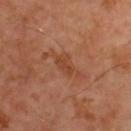<record>
  <biopsy_status>not biopsied; imaged during a skin examination</biopsy_status>
  <site>upper back</site>
  <automated_metrics>
    <area_mm2_approx>3.0</area_mm2_approx>
    <eccentricity>0.8</eccentricity>
    <shape_asymmetry>0.35</shape_asymmetry>
    <cielab_L>43</cielab_L>
    <cielab_a>25</cielab_a>
    <cielab_b>34</cielab_b>
    <vs_skin_darker_L>7.0</vs_skin_darker_L>
    <vs_skin_contrast_norm>6.5</vs_skin_contrast_norm>
    <border_irregularity_0_10>4.0</border_irregularity_0_10>
    <color_variation_0_10>0.0</color_variation_0_10>
  </automated_metrics>
  <patient>
    <sex>male</sex>
    <age_approx>50</age_approx>
  </patient>
  <lesion_size>
    <long_diameter_mm_approx>2.5</long_diameter_mm_approx>
  </lesion_size>
  <image>
    <source>total-body photography crop</source>
    <field_of_view_mm>15</field_of_view_mm>
  </image>
  <lighting>cross-polarized</lighting>
</record>